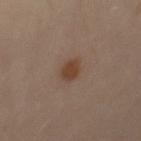| key | value |
|---|---|
| biopsy status | imaged on a skin check; not biopsied |
| TBP lesion metrics | a lesion–skin lightness drop of about 9 and a normalized border contrast of about 8; a nevus-likeness score of about 100/100 and a detector confidence of about 100 out of 100 that the crop contains a lesion |
| subject | female, aged around 40 |
| body site | the left leg |
| size | ≈2.5 mm |
| imaging modality | 15 mm crop, total-body photography |
| lighting | cross-polarized |